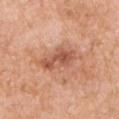  biopsy_status: not biopsied; imaged during a skin examination
  lighting: white-light
  image:
    source: total-body photography crop
    field_of_view_mm: 15
  site: left upper arm
  patient:
    sex: male
    age_approx: 60
  lesion_size:
    long_diameter_mm_approx: 4.5